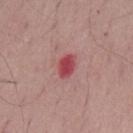workup = no biopsy performed (imaged during a skin exam); acquisition = total-body-photography crop, ~15 mm field of view; patient = male, aged around 65; site = the mid back.